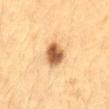  biopsy_status: not biopsied; imaged during a skin examination
  image:
    source: total-body photography crop
    field_of_view_mm: 15
  lesion_size:
    long_diameter_mm_approx: 3.0
  automated_metrics:
    eccentricity: 0.6
    shape_asymmetry: 0.15
    cielab_L: 55
    cielab_a: 20
    cielab_b: 38
    vs_skin_darker_L: 18.0
    vs_skin_contrast_norm: 11.5
    border_irregularity_0_10: 1.5
    color_variation_0_10: 5.0
    peripheral_color_asymmetry: 1.5
    lesion_detection_confidence_0_100: 100
  lighting: cross-polarized
  site: abdomen
  patient:
    sex: female
    age_approx: 30